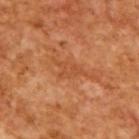Clinical impression:
The lesion was photographed on a routine skin check and not biopsied; there is no pathology result.
Context:
The lesion's longest dimension is about 3.5 mm. This image is a 15 mm lesion crop taken from a total-body photograph. The tile uses cross-polarized illumination. The subject is a male about 65 years old.